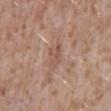The lesion was tiled from a total-body skin photograph and was not biopsied. A close-up tile cropped from a whole-body skin photograph, about 15 mm across. The lesion's longest dimension is about 2.5 mm. The total-body-photography lesion software estimated a footprint of about 4 mm², an outline eccentricity of about 0.75 (0 = round, 1 = elongated), and a symmetry-axis asymmetry near 0.3. The analysis additionally found a lesion color around L≈53 a*≈19 b*≈27 in CIELAB and a normalized lesion–skin contrast near 5. The software also gave a classifier nevus-likeness of about 0/100 and a detector confidence of about 85 out of 100 that the crop contains a lesion. A male patient aged 43 to 47. Located on the back. The tile uses white-light illumination.